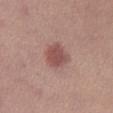biopsy status: catalogued during a skin exam; not biopsied | lesion diameter: ~3.5 mm (longest diameter) | lighting: white-light illumination | automated lesion analysis: a footprint of about 7.5 mm², an outline eccentricity of about 0.65 (0 = round, 1 = elongated), and a shape-asymmetry score of about 0.2 (0 = symmetric) | patient: female, aged around 25 | location: the left lower leg | imaging modality: 15 mm crop, total-body photography.